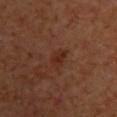follow-up: no biopsy performed (imaged during a skin exam)
subject: female, approximately 40 years of age
automated metrics: a lesion color around L≈28 a*≈22 b*≈28 in CIELAB, about 6 CIELAB-L* units darker than the surrounding skin, and a lesion-to-skin contrast of about 7 (normalized; higher = more distinct); an automated nevus-likeness rating near 5 out of 100 and a lesion-detection confidence of about 100/100
site: the upper back
lesion size: ~3 mm (longest diameter)
acquisition: ~15 mm tile from a whole-body skin photo